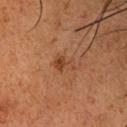Q: Was this lesion biopsied?
A: imaged on a skin check; not biopsied